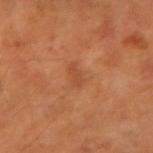workup — no biopsy performed (imaged during a skin exam); size — ~2.5 mm (longest diameter); imaging modality — 15 mm crop, total-body photography; body site — the left forearm; illumination — cross-polarized illumination; patient — male, in their 50s.